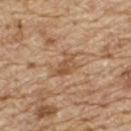| field | value |
|---|---|
| follow-up | total-body-photography surveillance lesion; no biopsy |
| diameter | about 3.5 mm |
| image source | total-body-photography crop, ~15 mm field of view |
| body site | the upper back |
| subject | male, in their 70s |